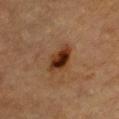Assessment:
Recorded during total-body skin imaging; not selected for excision or biopsy.
Background:
The lesion is located on the chest. A male patient, in their mid-80s or older. Captured under cross-polarized illumination. Cropped from a whole-body photographic skin survey; the tile spans about 15 mm. Approximately 4 mm at its widest.